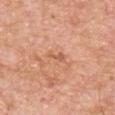Assessment:
Captured during whole-body skin photography for melanoma surveillance; the lesion was not biopsied.
Image and clinical context:
The lesion's longest dimension is about 2.5 mm. Cropped from a total-body skin-imaging series; the visible field is about 15 mm. The total-body-photography lesion software estimated an area of roughly 2.5 mm², a shape eccentricity near 0.9, and two-axis asymmetry of about 0.35. From the chest. Captured under white-light illumination. A male subject, aged approximately 55.